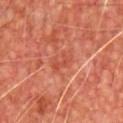notes: catalogued during a skin exam; not biopsied
lighting: cross-polarized illumination
image source: total-body-photography crop, ~15 mm field of view
body site: the front of the torso
subject: male, aged 63 to 67